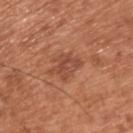Notes:
* biopsy status — total-body-photography surveillance lesion; no biopsy
* lighting — white-light
* site — the upper back
* subject — male, aged 53–57
* lesion size — about 4 mm
* image — 15 mm crop, total-body photography